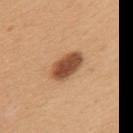Impression:
Recorded during total-body skin imaging; not selected for excision or biopsy.
Background:
A female subject aged 28 to 32. The tile uses white-light illumination. This image is a 15 mm lesion crop taken from a total-body photograph. An algorithmic analysis of the crop reported a lesion area of about 9.5 mm². And it measured a border-irregularity index near 2/10, internal color variation of about 4 on a 0–10 scale, and a peripheral color-asymmetry measure near 1. The lesion is located on the upper back.